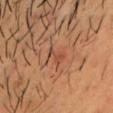A close-up tile cropped from a whole-body skin photograph, about 15 mm across. The lesion is on the chest. A male subject aged approximately 35. The lesion-visualizer software estimated a footprint of about 3.5 mm² and a shape-asymmetry score of about 0.5 (0 = symmetric). This is a cross-polarized tile. About 2.5 mm across.